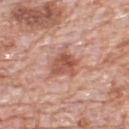Clinical summary: Cropped from a total-body skin-imaging series; the visible field is about 15 mm. The patient is a male aged approximately 80. The lesion is on the upper back. Captured under white-light illumination. Longest diameter approximately 4 mm.